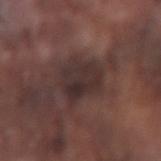Cropped from a total-body skin-imaging series; the visible field is about 15 mm. From the arm. Imaged with white-light lighting. A male subject aged approximately 75.